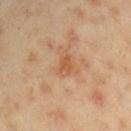<tbp_lesion>
<site>right upper arm</site>
<lighting>cross-polarized</lighting>
<patient>
  <sex>male</sex>
  <age_approx>45</age_approx>
</patient>
<image>
  <source>total-body photography crop</source>
  <field_of_view_mm>15</field_of_view_mm>
</image>
<lesion_size>
  <long_diameter_mm_approx>3.0</long_diameter_mm_approx>
</lesion_size>
</tbp_lesion>